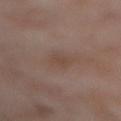This lesion was catalogued during total-body skin photography and was not selected for biopsy.
Cropped from a total-body skin-imaging series; the visible field is about 15 mm.
From the right thigh.
Automated image analysis of the tile measured an automated nevus-likeness rating near 0 out of 100.
Approximately 4 mm at its widest.
A female subject aged 53 to 57.